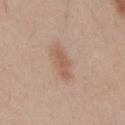Part of a total-body skin-imaging series; this lesion was reviewed on a skin check and was not flagged for biopsy. The lesion is located on the mid back. Imaged with white-light lighting. A roughly 15 mm field-of-view crop from a total-body skin photograph. A male subject in their 40s.